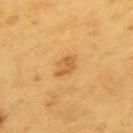Assessment:
The lesion was photographed on a routine skin check and not biopsied; there is no pathology result.
Clinical summary:
The lesion's longest dimension is about 3 mm. An algorithmic analysis of the crop reported a lesion color around L≈59 a*≈23 b*≈47 in CIELAB and a normalized lesion–skin contrast near 6. It also reported a nevus-likeness score of about 45/100 and a lesion-detection confidence of about 100/100. A male patient in their 60s. Cropped from a total-body skin-imaging series; the visible field is about 15 mm. From the left upper arm.